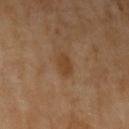Clinical impression: The lesion was photographed on a routine skin check and not biopsied; there is no pathology result. Image and clinical context: A female patient roughly 70 years of age. The lesion is on the left upper arm. An algorithmic analysis of the crop reported a mean CIELAB color near L≈42 a*≈19 b*≈33 and roughly 7 lightness units darker than nearby skin. The analysis additionally found an automated nevus-likeness rating near 15 out of 100 and a detector confidence of about 100 out of 100 that the crop contains a lesion. A lesion tile, about 15 mm wide, cut from a 3D total-body photograph. Imaged with cross-polarized lighting.